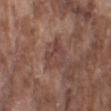This lesion was catalogued during total-body skin photography and was not selected for biopsy.
A male patient in their mid- to late 70s.
The lesion-visualizer software estimated a lesion color around L≈43 a*≈20 b*≈23 in CIELAB, a lesion–skin lightness drop of about 7, and a normalized border contrast of about 5.5. It also reported border irregularity of about 4.5 on a 0–10 scale and a color-variation rating of about 3.5/10. The software also gave a nevus-likeness score of about 0/100.
A close-up tile cropped from a whole-body skin photograph, about 15 mm across.
This is a white-light tile.
About 3.5 mm across.
The lesion is on the arm.